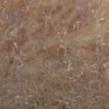workup=no biopsy performed (imaged during a skin exam)
patient=male, aged 83 or older
tile lighting=cross-polarized
body site=the right lower leg
image source=~15 mm crop, total-body skin-cancer survey